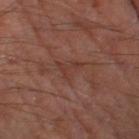| key | value |
|---|---|
| notes | catalogued during a skin exam; not biopsied |
| acquisition | 15 mm crop, total-body photography |
| anatomic site | the left thigh |
| illumination | cross-polarized illumination |
| size | about 3 mm |
| image-analysis metrics | a lesion color around L≈34 a*≈20 b*≈23 in CIELAB and about 5 CIELAB-L* units darker than the surrounding skin; a classifier nevus-likeness of about 0/100 |
| subject | male, aged approximately 55 |